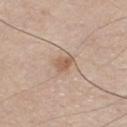Impression: No biopsy was performed on this lesion — it was imaged during a full skin examination and was not determined to be concerning. Clinical summary: Longest diameter approximately 2.5 mm. The lesion is on the chest. A male patient in their mid-40s. A lesion tile, about 15 mm wide, cut from a 3D total-body photograph.